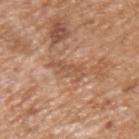acquisition: total-body-photography crop, ~15 mm field of view; anatomic site: the left upper arm; subject: male, in their mid- to late 60s.